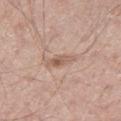The lesion-visualizer software estimated a footprint of about 4.5 mm² and an outline eccentricity of about 0.9 (0 = round, 1 = elongated). It also reported a mean CIELAB color near L≈58 a*≈18 b*≈27, about 9 CIELAB-L* units darker than the surrounding skin, and a normalized border contrast of about 6.5. And it measured a border-irregularity index near 3/10, internal color variation of about 4.5 on a 0–10 scale, and radial color variation of about 1.5. And it measured a detector confidence of about 100 out of 100 that the crop contains a lesion. Cropped from a whole-body photographic skin survey; the tile spans about 15 mm. Captured under white-light illumination. The subject is a male aged 53–57. The lesion is on the leg. Measured at roughly 3.5 mm in maximum diameter.